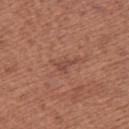  patient:
    sex: female
    age_approx: 50
  site: upper back
  image:
    source: total-body photography crop
    field_of_view_mm: 15
  lighting: white-light
  automated_metrics:
    area_mm2_approx: 3.0
    eccentricity: 0.85
    shape_asymmetry: 0.6
    nevus_likeness_0_100: 0